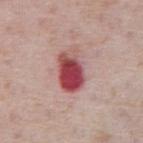{
  "biopsy_status": "not biopsied; imaged during a skin examination",
  "patient": {
    "sex": "male",
    "age_approx": 75
  },
  "automated_metrics": {
    "border_irregularity_0_10": 2.0,
    "color_variation_0_10": 10.0,
    "nevus_likeness_0_100": 0,
    "lesion_detection_confidence_0_100": 100
  },
  "image": {
    "source": "total-body photography crop",
    "field_of_view_mm": 15
  },
  "site": "abdomen",
  "lesion_size": {
    "long_diameter_mm_approx": 5.0
  },
  "lighting": "white-light"
}